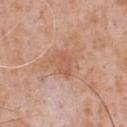Impression: The lesion was tiled from a total-body skin photograph and was not biopsied. Acquisition and patient details: A male subject, roughly 65 years of age. Cropped from a whole-body photographic skin survey; the tile spans about 15 mm. About 3 mm across. Captured under white-light illumination. On the front of the torso.